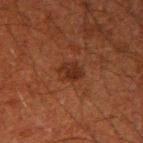workup — imaged on a skin check; not biopsied.